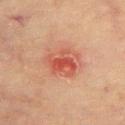Clinical impression: Imaged during a routine full-body skin examination; the lesion was not biopsied and no histopathology is available. Image and clinical context: The subject is a male aged 73–77. The lesion is on the upper back. Imaged with cross-polarized lighting. Automated image analysis of the tile measured a footprint of about 11 mm², an outline eccentricity of about 0.7 (0 = round, 1 = elongated), and a shape-asymmetry score of about 0.25 (0 = symmetric). A 15 mm crop from a total-body photograph taken for skin-cancer surveillance. The recorded lesion diameter is about 4.5 mm.